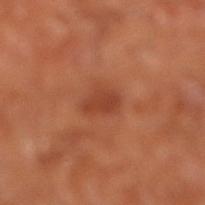Impression:
Imaged during a routine full-body skin examination; the lesion was not biopsied and no histopathology is available.
Background:
A roughly 15 mm field-of-view crop from a total-body skin photograph. The subject is aged 63–67. The lesion is on the right lower leg. Approximately 3 mm at its widest.